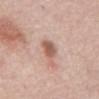Clinical impression: The lesion was tiled from a total-body skin photograph and was not biopsied. Acquisition and patient details: Imaged with white-light lighting. The total-body-photography lesion software estimated a normalized border contrast of about 8.5. The software also gave border irregularity of about 2 on a 0–10 scale, a color-variation rating of about 3/10, and a peripheral color-asymmetry measure near 1. A close-up tile cropped from a whole-body skin photograph, about 15 mm across. The recorded lesion diameter is about 3 mm. Located on the abdomen. The subject is a female aged 63–67.